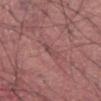follow-up: total-body-photography surveillance lesion; no biopsy | lesion size: ~2.5 mm (longest diameter) | image: ~15 mm tile from a whole-body skin photo | site: the left thigh | subject: male, aged 38–42.